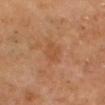Recorded during total-body skin imaging; not selected for excision or biopsy. The lesion is located on the head or neck. Measured at roughly 2.5 mm in maximum diameter. A close-up tile cropped from a whole-body skin photograph, about 15 mm across. The subject is a male in their 60s.